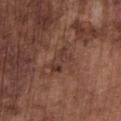• workup · no biopsy performed (imaged during a skin exam)
• location · the chest
• image source · total-body-photography crop, ~15 mm field of view
• automated metrics · a lesion area of about 4.5 mm², a shape eccentricity near 0.85, and a symmetry-axis asymmetry near 0.35; a lesion color around L≈36 a*≈20 b*≈23 in CIELAB, roughly 6 lightness units darker than nearby skin, and a normalized lesion–skin contrast near 6; a border-irregularity rating of about 5/10 and radial color variation of about 0.5; a lesion-detection confidence of about 95/100
• illumination · white-light illumination
• patient · male, in their mid- to late 70s